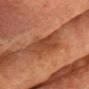Imaged during a routine full-body skin examination; the lesion was not biopsied and no histopathology is available. Located on the chest. The recorded lesion diameter is about 3.5 mm. A region of skin cropped from a whole-body photographic capture, roughly 15 mm wide. A male patient roughly 75 years of age.